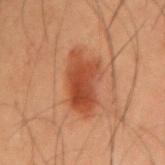Part of a total-body skin-imaging series; this lesion was reviewed on a skin check and was not flagged for biopsy. The patient is a male about 55 years old. From the right upper arm. A 15 mm close-up tile from a total-body photography series done for melanoma screening.